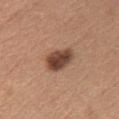This image is a 15 mm lesion crop taken from a total-body photograph. On the chest. Captured under white-light illumination. A female patient, aged 43 to 47. About 4 mm across.